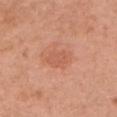Q: Is there a histopathology result?
A: total-body-photography surveillance lesion; no biopsy
Q: What kind of image is this?
A: total-body-photography crop, ~15 mm field of view
Q: Who is the patient?
A: female, aged 58–62
Q: Where on the body is the lesion?
A: the head or neck